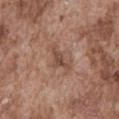| key | value |
|---|---|
| notes | catalogued during a skin exam; not biopsied |
| image source | ~15 mm crop, total-body skin-cancer survey |
| patient | male, about 75 years old |
| illumination | white-light illumination |
| body site | the abdomen |